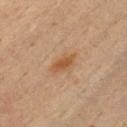Captured during whole-body skin photography for melanoma surveillance; the lesion was not biopsied. On the chest. This is a cross-polarized tile. Approximately 3.5 mm at its widest. The lesion-visualizer software estimated a lesion area of about 4.5 mm², a shape eccentricity near 0.85, and two-axis asymmetry of about 0.25. The software also gave an automated nevus-likeness rating near 80 out of 100 and lesion-presence confidence of about 100/100. A male patient aged 48–52. Cropped from a whole-body photographic skin survey; the tile spans about 15 mm.